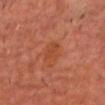follow-up = imaged on a skin check; not biopsied
patient = male, about 65 years old
illumination = cross-polarized
imaging modality = 15 mm crop, total-body photography
size = about 3.5 mm
body site = the upper back